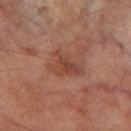Findings:
* lesion diameter — ~4 mm (longest diameter)
* tile lighting — cross-polarized
* acquisition — total-body-photography crop, ~15 mm field of view
* automated metrics — a lesion color around L≈43 a*≈23 b*≈28 in CIELAB, roughly 7 lightness units darker than nearby skin, and a lesion-to-skin contrast of about 6 (normalized; higher = more distinct); border irregularity of about 5.5 on a 0–10 scale and internal color variation of about 4.5 on a 0–10 scale; a nevus-likeness score of about 0/100 and a detector confidence of about 100 out of 100 that the crop contains a lesion
* body site — the leg
* patient — male, in their 70s